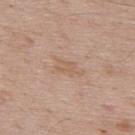Q: Was a biopsy performed?
A: catalogued during a skin exam; not biopsied
Q: Patient demographics?
A: male, about 70 years old
Q: What kind of image is this?
A: ~15 mm crop, total-body skin-cancer survey
Q: Lesion location?
A: the back
Q: How was the tile lit?
A: white-light illumination
Q: What is the lesion's diameter?
A: ~3.5 mm (longest diameter)